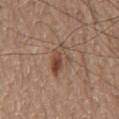Impression: Part of a total-body skin-imaging series; this lesion was reviewed on a skin check and was not flagged for biopsy. Image and clinical context: A male patient aged approximately 80. A lesion tile, about 15 mm wide, cut from a 3D total-body photograph. This is a white-light tile. The lesion is located on the front of the torso.